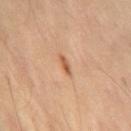Assessment:
The lesion was tiled from a total-body skin photograph and was not biopsied.
Context:
About 2.5 mm across. A male patient aged 53–57. A 15 mm close-up extracted from a 3D total-body photography capture. On the lower back. Captured under cross-polarized illumination.